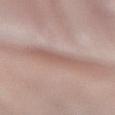The lesion is located on the left forearm.
Captured under white-light illumination.
The subject is a male aged 73 to 77.
This image is a 15 mm lesion crop taken from a total-body photograph.
The total-body-photography lesion software estimated roughly 8 lightness units darker than nearby skin. It also reported a border-irregularity rating of about 4/10, internal color variation of about 2.5 on a 0–10 scale, and peripheral color asymmetry of about 1.
Measured at roughly 5 mm in maximum diameter.